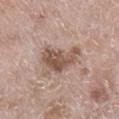Clinical impression: This lesion was catalogued during total-body skin photography and was not selected for biopsy. Acquisition and patient details: The tile uses white-light illumination. A close-up tile cropped from a whole-body skin photograph, about 15 mm across. A female subject, aged 73–77. Longest diameter approximately 5.5 mm. The lesion is located on the right lower leg. An algorithmic analysis of the crop reported a mean CIELAB color near L≈53 a*≈18 b*≈25 and a lesion–skin lightness drop of about 12.